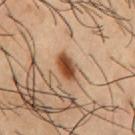<case>
<biopsy_status>not biopsied; imaged during a skin examination</biopsy_status>
<lighting>cross-polarized</lighting>
<patient>
  <sex>male</sex>
  <age_approx>55</age_approx>
</patient>
<lesion_size>
  <long_diameter_mm_approx>4.0</long_diameter_mm_approx>
</lesion_size>
<image>
  <source>total-body photography crop</source>
  <field_of_view_mm>15</field_of_view_mm>
</image>
<site>chest</site>
<automated_metrics>
  <area_mm2_approx>7.0</area_mm2_approx>
  <eccentricity>0.8</eccentricity>
  <shape_asymmetry>0.2</shape_asymmetry>
  <border_irregularity_0_10>2.0</border_irregularity_0_10>
  <color_variation_0_10>6.5</color_variation_0_10>
  <peripheral_color_asymmetry>2.0</peripheral_color_asymmetry>
  <nevus_likeness_0_100>100</nevus_likeness_0_100>
  <lesion_detection_confidence_0_100>100</lesion_detection_confidence_0_100>
</automated_metrics>
</case>